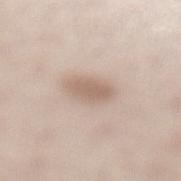The tile uses white-light illumination. Longest diameter approximately 3.5 mm. The lesion is located on the left lower leg. A region of skin cropped from a whole-body photographic capture, roughly 15 mm wide. The patient is a female aged around 40.